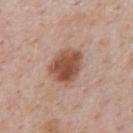Assessment:
No biopsy was performed on this lesion — it was imaged during a full skin examination and was not determined to be concerning.
Context:
The lesion-visualizer software estimated a footprint of about 12 mm² and an eccentricity of roughly 0.6. Longest diameter approximately 4.5 mm. The subject is a male aged around 50. The tile uses white-light illumination. A 15 mm crop from a total-body photograph taken for skin-cancer surveillance. On the mid back.